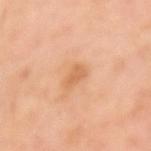<lesion>
<biopsy_status>not biopsied; imaged during a skin examination</biopsy_status>
<image>
  <source>total-body photography crop</source>
  <field_of_view_mm>15</field_of_view_mm>
</image>
<site>left upper arm</site>
<patient>
  <sex>female</sex>
  <age_approx>55</age_approx>
</patient>
<lighting>cross-polarized</lighting>
</lesion>